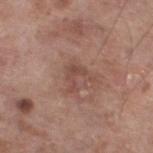<case>
<biopsy_status>not biopsied; imaged during a skin examination</biopsy_status>
<patient>
  <sex>male</sex>
  <age_approx>80</age_approx>
</patient>
<image>
  <source>total-body photography crop</source>
  <field_of_view_mm>15</field_of_view_mm>
</image>
<site>left lower leg</site>
<lesion_size>
  <long_diameter_mm_approx>3.0</long_diameter_mm_approx>
</lesion_size>
<lighting>white-light</lighting>
<automated_metrics>
  <nevus_likeness_0_100>0</nevus_likeness_0_100>
</automated_metrics>
</case>